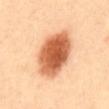  biopsy_status: not biopsied; imaged during a skin examination
  lighting: cross-polarized
  image:
    source: total-body photography crop
    field_of_view_mm: 15
  site: abdomen
  patient:
    sex: male
    age_approx: 40